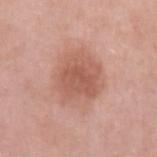This lesion was catalogued during total-body skin photography and was not selected for biopsy. Captured under white-light illumination. From the left upper arm. A 15 mm close-up extracted from a 3D total-body photography capture. The subject is a female about 45 years old. The lesion-visualizer software estimated a mean CIELAB color near L≈58 a*≈24 b*≈29, about 10 CIELAB-L* units darker than the surrounding skin, and a normalized lesion–skin contrast near 6.5. It also reported an automated nevus-likeness rating near 15 out of 100 and a detector confidence of about 100 out of 100 that the crop contains a lesion.